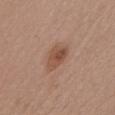Assessment:
Recorded during total-body skin imaging; not selected for excision or biopsy.
Background:
From the chest. An algorithmic analysis of the crop reported a footprint of about 7 mm² and two-axis asymmetry of about 0.25. The analysis additionally found a mean CIELAB color near L≈50 a*≈21 b*≈29 and a normalized border contrast of about 7. And it measured border irregularity of about 2.5 on a 0–10 scale, a color-variation rating of about 5.5/10, and a peripheral color-asymmetry measure near 2. This image is a 15 mm lesion crop taken from a total-body photograph. A female patient aged around 35. About 3.5 mm across.